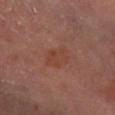Assessment: Recorded during total-body skin imaging; not selected for excision or biopsy. Clinical summary: Captured under cross-polarized illumination. A male patient, aged 63–67. A 15 mm close-up tile from a total-body photography series done for melanoma screening. The lesion is on the right lower leg. About 3.5 mm across. The total-body-photography lesion software estimated a shape eccentricity near 0.65 and two-axis asymmetry of about 0.2. It also reported a color-variation rating of about 3/10 and radial color variation of about 1. It also reported a lesion-detection confidence of about 100/100.